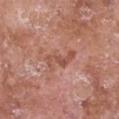Approximately 4 mm at its widest.
The total-body-photography lesion software estimated a lesion area of about 6 mm², a shape eccentricity near 0.9, and a symmetry-axis asymmetry near 0.55. The software also gave a border-irregularity index near 7/10, internal color variation of about 2 on a 0–10 scale, and peripheral color asymmetry of about 0.5. The analysis additionally found a classifier nevus-likeness of about 0/100 and a lesion-detection confidence of about 100/100.
A close-up tile cropped from a whole-body skin photograph, about 15 mm across.
From the chest.
The tile uses white-light illumination.
The subject is a female roughly 75 years of age.